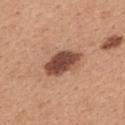workup = catalogued during a skin exam; not biopsied
patient = female, aged 28 to 32
body site = the upper back
lesion size = ~5 mm (longest diameter)
lighting = white-light illumination
image = ~15 mm tile from a whole-body skin photo